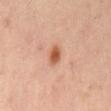workup: catalogued during a skin exam; not biopsied
acquisition: ~15 mm tile from a whole-body skin photo
lesion size: about 3 mm
TBP lesion metrics: an automated nevus-likeness rating near 95 out of 100 and a detector confidence of about 100 out of 100 that the crop contains a lesion
subject: male, roughly 55 years of age
location: the mid back